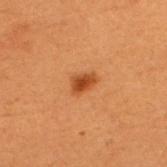• biopsy status · total-body-photography surveillance lesion; no biopsy
• site · the back
• subject · male, aged 48–52
• image · total-body-photography crop, ~15 mm field of view